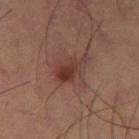follow-up = imaged on a skin check; not biopsied
body site = the left thigh
image source = 15 mm crop, total-body photography